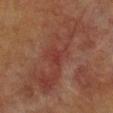Q: Was this lesion biopsied?
A: total-body-photography surveillance lesion; no biopsy
Q: What are the patient's age and sex?
A: female, aged approximately 40
Q: Lesion location?
A: the chest
Q: How was this image acquired?
A: total-body-photography crop, ~15 mm field of view
Q: What lighting was used for the tile?
A: cross-polarized illumination